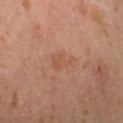<case>
  <biopsy_status>not biopsied; imaged during a skin examination</biopsy_status>
  <site>leg</site>
  <automated_metrics>
    <area_mm2_approx>5.0</area_mm2_approx>
    <eccentricity>0.85</eccentricity>
    <shape_asymmetry>0.45</shape_asymmetry>
    <cielab_L>53</cielab_L>
    <cielab_a>22</cielab_a>
    <cielab_b>32</cielab_b>
    <vs_skin_darker_L>5.0</vs_skin_darker_L>
    <vs_skin_contrast_norm>4.5</vs_skin_contrast_norm>
  </automated_metrics>
  <lesion_size>
    <long_diameter_mm_approx>3.5</long_diameter_mm_approx>
  </lesion_size>
  <patient>
    <sex>female</sex>
    <age_approx>40</age_approx>
  </patient>
  <image>
    <source>total-body photography crop</source>
    <field_of_view_mm>15</field_of_view_mm>
  </image>
</case>